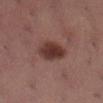patient=female, in their mid-50s
anatomic site=the leg
tile lighting=white-light illumination
lesion size=about 4 mm
TBP lesion metrics=a border-irregularity index near 1.5/10, a within-lesion color-variation index near 4/10, and a peripheral color-asymmetry measure near 1; a nevus-likeness score of about 95/100
image=total-body-photography crop, ~15 mm field of view